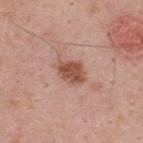On the upper back.
This image is a 15 mm lesion crop taken from a total-body photograph.
A male patient aged 43–47.